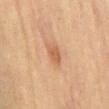Assessment: Captured during whole-body skin photography for melanoma surveillance; the lesion was not biopsied. Clinical summary: The patient is a male aged around 70. On the abdomen. This is a cross-polarized tile. This image is a 15 mm lesion crop taken from a total-body photograph. About 2.5 mm across.